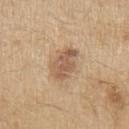Findings:
* patient — male, aged around 70
* image source — ~15 mm tile from a whole-body skin photo
* size — ≈5 mm
* tile lighting — white-light illumination
* anatomic site — the left upper arm
* automated lesion analysis — a mean CIELAB color near L≈58 a*≈17 b*≈32 and a normalized lesion–skin contrast near 7.5; a border-irregularity index near 2.5/10 and a within-lesion color-variation index near 4.5/10; a classifier nevus-likeness of about 45/100 and lesion-presence confidence of about 100/100